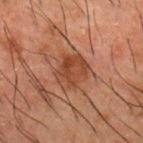workup: imaged on a skin check; not biopsied
patient: male, in their 50s
image: ~15 mm tile from a whole-body skin photo
lesion diameter: ~4.5 mm (longest diameter)
image-analysis metrics: an average lesion color of about L≈34 a*≈20 b*≈27 (CIELAB), roughly 7 lightness units darker than nearby skin, and a normalized lesion–skin contrast near 7
illumination: cross-polarized illumination
location: the back